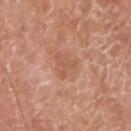Clinical impression: The lesion was photographed on a routine skin check and not biopsied; there is no pathology result. Clinical summary: The subject is a male in their mid- to late 60s. The recorded lesion diameter is about 3 mm. An algorithmic analysis of the crop reported a footprint of about 6 mm². The software also gave a mean CIELAB color near L≈56 a*≈23 b*≈32, a lesion–skin lightness drop of about 6, and a normalized border contrast of about 4.5. It also reported border irregularity of about 4 on a 0–10 scale, a color-variation rating of about 1.5/10, and radial color variation of about 0.5. The lesion is located on the right upper arm. Captured under white-light illumination. A close-up tile cropped from a whole-body skin photograph, about 15 mm across.